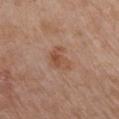| key | value |
|---|---|
| notes | imaged on a skin check; not biopsied |
| tile lighting | white-light illumination |
| location | the front of the torso |
| TBP lesion metrics | a footprint of about 7 mm² and a shape-asymmetry score of about 0.35 (0 = symmetric); a lesion color around L≈51 a*≈21 b*≈30 in CIELAB and a normalized border contrast of about 6.5; a border-irregularity rating of about 3.5/10, internal color variation of about 5 on a 0–10 scale, and radial color variation of about 1.5; an automated nevus-likeness rating near 15 out of 100 and a lesion-detection confidence of about 100/100 |
| subject | female, roughly 80 years of age |
| lesion diameter | about 3.5 mm |
| imaging modality | 15 mm crop, total-body photography |